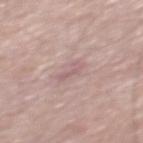| key | value |
|---|---|
| patient | male, aged approximately 60 |
| lesion size | ≈2.5 mm |
| acquisition | total-body-photography crop, ~15 mm field of view |
| site | the mid back |
| illumination | white-light illumination |